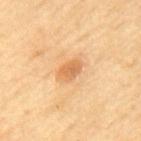Clinical impression: This lesion was catalogued during total-body skin photography and was not selected for biopsy. Context: The lesion's longest dimension is about 3 mm. This is a cross-polarized tile. A 15 mm close-up extracted from a 3D total-body photography capture. A male patient aged approximately 70. From the upper back.